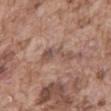* biopsy status — imaged on a skin check; not biopsied
* anatomic site — the abdomen
* patient — male, approximately 75 years of age
* illumination — white-light illumination
* lesion diameter — ≈4.5 mm
* image source — ~15 mm crop, total-body skin-cancer survey